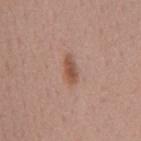Clinical impression: This lesion was catalogued during total-body skin photography and was not selected for biopsy. Clinical summary: Located on the mid back. Longest diameter approximately 3 mm. This is a white-light tile. An algorithmic analysis of the crop reported an outline eccentricity of about 0.85 (0 = round, 1 = elongated) and two-axis asymmetry of about 0.25. The software also gave a lesion–skin lightness drop of about 10 and a lesion-to-skin contrast of about 8 (normalized; higher = more distinct). The analysis additionally found a border-irregularity index near 2.5/10, a color-variation rating of about 3/10, and a peripheral color-asymmetry measure near 1. Cropped from a total-body skin-imaging series; the visible field is about 15 mm. A female subject, in their mid- to late 40s.